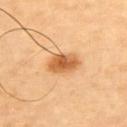Q: Was a biopsy performed?
A: catalogued during a skin exam; not biopsied
Q: Where on the body is the lesion?
A: the upper back
Q: Lesion size?
A: ≈4 mm
Q: What is the imaging modality?
A: 15 mm crop, total-body photography
Q: What did automated image analysis measure?
A: a lesion area of about 8 mm² and a symmetry-axis asymmetry near 0.15; an average lesion color of about L≈63 a*≈26 b*≈45 (CIELAB), a lesion–skin lightness drop of about 15, and a normalized border contrast of about 9
Q: Illumination type?
A: cross-polarized